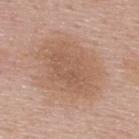workup: no biopsy performed (imaged during a skin exam) | subject: male, aged approximately 55 | tile lighting: white-light | lesion size: ≈6.5 mm | body site: the upper back | imaging modality: total-body-photography crop, ~15 mm field of view | image-analysis metrics: an average lesion color of about L≈57 a*≈19 b*≈30 (CIELAB) and a lesion-to-skin contrast of about 5 (normalized; higher = more distinct).